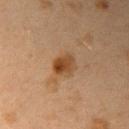Clinical impression: Recorded during total-body skin imaging; not selected for excision or biopsy. Context: A 15 mm close-up extracted from a 3D total-body photography capture. The patient is a female about 40 years old. The lesion's longest dimension is about 3 mm. On the right upper arm. This is a cross-polarized tile.